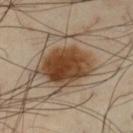  lesion_size:
    long_diameter_mm_approx: 5.5
  site: left thigh
  image:
    source: total-body photography crop
    field_of_view_mm: 15
  patient:
    sex: male
    age_approx: 55